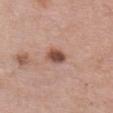notes: total-body-photography surveillance lesion; no biopsy
acquisition: ~15 mm tile from a whole-body skin photo
automated metrics: an average lesion color of about L≈49 a*≈22 b*≈26 (CIELAB) and roughly 15 lightness units darker than nearby skin
lesion diameter: about 2.5 mm
patient: female, in their mid- to late 60s
illumination: white-light illumination
location: the chest